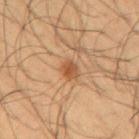A lesion tile, about 15 mm wide, cut from a 3D total-body photograph.
A male patient, roughly 55 years of age.
This is a cross-polarized tile.
The lesion is located on the right upper arm.
Longest diameter approximately 2.5 mm.